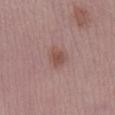Imaged during a routine full-body skin examination; the lesion was not biopsied and no histopathology is available. The tile uses white-light illumination. A 15 mm close-up tile from a total-body photography series done for melanoma screening. Automated tile analysis of the lesion measured an outline eccentricity of about 0.55 (0 = round, 1 = elongated). It also reported a border-irregularity rating of about 3/10 and internal color variation of about 1.5 on a 0–10 scale. The analysis additionally found a detector confidence of about 100 out of 100 that the crop contains a lesion. The subject is a male aged approximately 50. On the right lower leg.